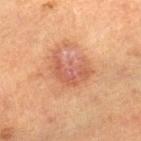Imaged during a routine full-body skin examination; the lesion was not biopsied and no histopathology is available.
An algorithmic analysis of the crop reported an area of roughly 12 mm², an eccentricity of roughly 0.7, and a symmetry-axis asymmetry near 0.4. The analysis additionally found a lesion color around L≈55 a*≈26 b*≈32 in CIELAB, roughly 9 lightness units darker than nearby skin, and a lesion-to-skin contrast of about 6.5 (normalized; higher = more distinct).
Cropped from a total-body skin-imaging series; the visible field is about 15 mm.
The patient is a female aged 58–62.
About 5 mm across.
From the right lower leg.